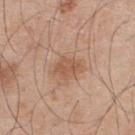Recorded during total-body skin imaging; not selected for excision or biopsy.
From the back.
The patient is a male about 55 years old.
A 15 mm crop from a total-body photograph taken for skin-cancer surveillance.